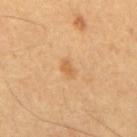| field | value |
|---|---|
| tile lighting | cross-polarized |
| lesion diameter | ≈2.5 mm |
| patient | male, about 60 years old |
| image-analysis metrics | a footprint of about 3 mm² and an outline eccentricity of about 0.8 (0 = round, 1 = elongated); a lesion color around L≈57 a*≈19 b*≈38 in CIELAB, a lesion–skin lightness drop of about 7, and a lesion-to-skin contrast of about 5.5 (normalized; higher = more distinct); a classifier nevus-likeness of about 15/100 and lesion-presence confidence of about 100/100 |
| image source | ~15 mm tile from a whole-body skin photo |
| location | the mid back |